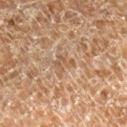Assessment:
Part of a total-body skin-imaging series; this lesion was reviewed on a skin check and was not flagged for biopsy.
Context:
A male subject, about 60 years old. From the right lower leg. About 2.5 mm across. The total-body-photography lesion software estimated border irregularity of about 5.5 on a 0–10 scale, a color-variation rating of about 0/10, and radial color variation of about 0. The software also gave an automated nevus-likeness rating near 0 out of 100 and a detector confidence of about 70 out of 100 that the crop contains a lesion. Cropped from a total-body skin-imaging series; the visible field is about 15 mm. The tile uses cross-polarized illumination.